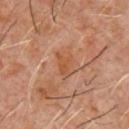Notes:
- notes — no biopsy performed (imaged during a skin exam)
- acquisition — ~15 mm tile from a whole-body skin photo
- body site — the chest
- patient — male, aged 58 to 62
- tile lighting — cross-polarized illumination